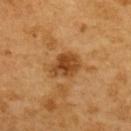Q: Was a biopsy performed?
A: no biopsy performed (imaged during a skin exam)
Q: How large is the lesion?
A: ≈3.5 mm
Q: Illumination type?
A: cross-polarized
Q: What is the anatomic site?
A: the upper back
Q: Automated lesion metrics?
A: a lesion area of about 8.5 mm² and two-axis asymmetry of about 0.25
Q: What kind of image is this?
A: ~15 mm crop, total-body skin-cancer survey
Q: Patient demographics?
A: female, in their mid- to late 50s